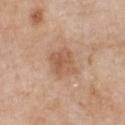Assessment:
Part of a total-body skin-imaging series; this lesion was reviewed on a skin check and was not flagged for biopsy.
Background:
Longest diameter approximately 3.5 mm. This is a white-light tile. A 15 mm close-up extracted from a 3D total-body photography capture. A female patient, roughly 40 years of age. The lesion is located on the front of the torso.